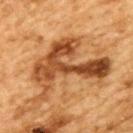Recorded during total-body skin imaging; not selected for excision or biopsy.
A 15 mm close-up extracted from a 3D total-body photography capture.
Imaged with cross-polarized lighting.
On the back.
A male patient, aged approximately 85 or older.
About 10 mm across.
Automated image analysis of the tile measured a lesion color around L≈42 a*≈23 b*≈36 in CIELAB, a lesion–skin lightness drop of about 13, and a normalized lesion–skin contrast near 10. The software also gave internal color variation of about 8.5 on a 0–10 scale and peripheral color asymmetry of about 3.